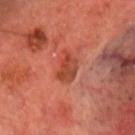follow-up — total-body-photography surveillance lesion; no biopsy | imaging modality — 15 mm crop, total-body photography | anatomic site — the head or neck | automated metrics — a footprint of about 6.5 mm², a shape eccentricity near 0.7, and two-axis asymmetry of about 0.3; about 9 CIELAB-L* units darker than the surrounding skin and a lesion-to-skin contrast of about 7 (normalized; higher = more distinct); a border-irregularity index near 3/10, a color-variation rating of about 4/10, and peripheral color asymmetry of about 1.5 | patient — male, approximately 70 years of age | lesion size — about 3.5 mm | illumination — cross-polarized illumination.